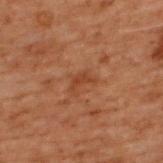• workup · imaged on a skin check; not biopsied
• image · ~15 mm crop, total-body skin-cancer survey
• site · the upper back
• automated metrics · a mean CIELAB color near L≈32 a*≈20 b*≈28 and a lesion-to-skin contrast of about 5.5 (normalized; higher = more distinct); a border-irregularity index near 5.5/10 and a within-lesion color-variation index near 0.5/10
• patient · male, aged 68 to 72
• lighting · cross-polarized illumination
• diameter · ≈2.5 mm